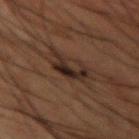biopsy_status: not biopsied; imaged during a skin examination
patient:
  sex: male
  age_approx: 50
automated_metrics:
  area_mm2_approx: 6.0
  eccentricity: 0.65
  shape_asymmetry: 0.55
  cielab_L: 18
  cielab_a: 11
  cielab_b: 17
  vs_skin_darker_L: 8.0
  vs_skin_contrast_norm: 10.5
  nevus_likeness_0_100: 50
site: right forearm
image:
  source: total-body photography crop
  field_of_view_mm: 15
lesion_size:
  long_diameter_mm_approx: 3.5